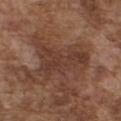This is a white-light tile. The subject is a male about 75 years old. From the chest. A roughly 15 mm field-of-view crop from a total-body skin photograph.